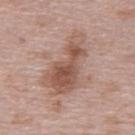Q: Was this lesion biopsied?
A: imaged on a skin check; not biopsied
Q: How was this image acquired?
A: ~15 mm tile from a whole-body skin photo
Q: Automated lesion metrics?
A: an average lesion color of about L≈54 a*≈20 b*≈26 (CIELAB), a lesion–skin lightness drop of about 11, and a normalized lesion–skin contrast near 8
Q: Patient demographics?
A: female, aged around 65
Q: What is the anatomic site?
A: the upper back